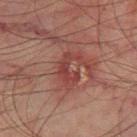{
  "biopsy_status": "not biopsied; imaged during a skin examination",
  "automated_metrics": {
    "area_mm2_approx": 5.0,
    "eccentricity": 0.8,
    "shape_asymmetry": 0.65,
    "cielab_L": 34,
    "cielab_a": 25,
    "cielab_b": 21,
    "vs_skin_darker_L": 7.0,
    "vs_skin_contrast_norm": 6.5,
    "border_irregularity_0_10": 8.5,
    "color_variation_0_10": 0.0,
    "nevus_likeness_0_100": 0
  },
  "lesion_size": {
    "long_diameter_mm_approx": 3.5
  },
  "site": "right thigh",
  "image": {
    "source": "total-body photography crop",
    "field_of_view_mm": 15
  },
  "lighting": "cross-polarized",
  "patient": {
    "sex": "male",
    "age_approx": 75
  }
}